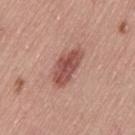| field | value |
|---|---|
| biopsy status | imaged on a skin check; not biopsied |
| patient | female, aged around 50 |
| lesion diameter | ≈5 mm |
| acquisition | 15 mm crop, total-body photography |
| anatomic site | the left thigh |
| illumination | white-light illumination |
| automated lesion analysis | a footprint of about 10 mm², an outline eccentricity of about 0.9 (0 = round, 1 = elongated), and two-axis asymmetry of about 0.25; an average lesion color of about L≈51 a*≈25 b*≈26 (CIELAB) and a normalized lesion–skin contrast near 9; a nevus-likeness score of about 90/100 and a lesion-detection confidence of about 100/100 |